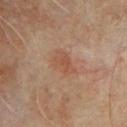Part of a total-body skin-imaging series; this lesion was reviewed on a skin check and was not flagged for biopsy. The tile uses cross-polarized illumination. The patient is a male about 65 years old. The lesion's longest dimension is about 3.5 mm. Cropped from a whole-body photographic skin survey; the tile spans about 15 mm. The lesion is on the chest.